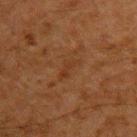Recorded during total-body skin imaging; not selected for excision or biopsy.
This is a cross-polarized tile.
Cropped from a total-body skin-imaging series; the visible field is about 15 mm.
On the left upper arm.
The patient is a male in their 60s.
The recorded lesion diameter is about 3.5 mm.
Automated tile analysis of the lesion measured an eccentricity of roughly 0.9 and a symmetry-axis asymmetry near 0.45. And it measured about 4 CIELAB-L* units darker than the surrounding skin and a normalized lesion–skin contrast near 4.5. The analysis additionally found a border-irregularity rating of about 5.5/10, a color-variation rating of about 1/10, and peripheral color asymmetry of about 0.5. The analysis additionally found a nevus-likeness score of about 0/100 and a detector confidence of about 90 out of 100 that the crop contains a lesion.